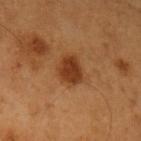Q: Was this lesion biopsied?
A: catalogued during a skin exam; not biopsied
Q: What is the lesion's diameter?
A: ~3.5 mm (longest diameter)
Q: What lighting was used for the tile?
A: cross-polarized illumination
Q: Who is the patient?
A: male, in their 60s
Q: What did automated image analysis measure?
A: border irregularity of about 2.5 on a 0–10 scale, a color-variation rating of about 2.5/10, and a peripheral color-asymmetry measure near 1
Q: Lesion location?
A: the left upper arm
Q: What kind of image is this?
A: total-body-photography crop, ~15 mm field of view